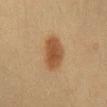<lesion>
  <biopsy_status>not biopsied; imaged during a skin examination</biopsy_status>
  <lesion_size>
    <long_diameter_mm_approx>4.5</long_diameter_mm_approx>
  </lesion_size>
  <site>chest</site>
  <patient>
    <sex>female</sex>
    <age_approx>30</age_approx>
  </patient>
  <image>
    <source>total-body photography crop</source>
    <field_of_view_mm>15</field_of_view_mm>
  </image>
  <automated_metrics>
    <shape_asymmetry>0.2</shape_asymmetry>
    <border_irregularity_0_10>2.0</border_irregularity_0_10>
    <peripheral_color_asymmetry>1.0</peripheral_color_asymmetry>
  </automated_metrics>
  <lighting>cross-polarized</lighting>
</lesion>